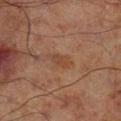notes=imaged on a skin check; not biopsied
body site=the leg
image source=~15 mm crop, total-body skin-cancer survey
patient=male, about 70 years old